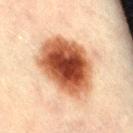Captured during whole-body skin photography for melanoma surveillance; the lesion was not biopsied. Automated tile analysis of the lesion measured a mean CIELAB color near L≈45 a*≈23 b*≈31, roughly 21 lightness units darker than nearby skin, and a normalized lesion–skin contrast near 14.5. And it measured a within-lesion color-variation index near 8/10 and radial color variation of about 2. The software also gave a nevus-likeness score of about 100/100 and a detector confidence of about 100 out of 100 that the crop contains a lesion. A roughly 15 mm field-of-view crop from a total-body skin photograph. Approximately 7.5 mm at its widest. Captured under cross-polarized illumination. On the right thigh. The patient is a male approximately 40 years of age.